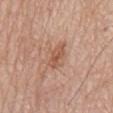Imaged during a routine full-body skin examination; the lesion was not biopsied and no histopathology is available.
A roughly 15 mm field-of-view crop from a total-body skin photograph.
The lesion is located on the mid back.
A male subject aged 78 to 82.